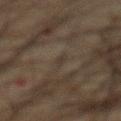Case summary:
* notes: total-body-photography surveillance lesion; no biopsy
* subject: male, aged 63 to 67
* illumination: cross-polarized illumination
* automated metrics: a lesion area of about 1 mm², an outline eccentricity of about 0.9 (0 = round, 1 = elongated), and a symmetry-axis asymmetry near 0.35; peripheral color asymmetry of about 0; a nevus-likeness score of about 0/100
* image: total-body-photography crop, ~15 mm field of view
* anatomic site: the abdomen